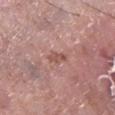Notes:
- biopsy status: total-body-photography surveillance lesion; no biopsy
- automated lesion analysis: a lesion color around L≈52 a*≈23 b*≈25 in CIELAB, roughly 9 lightness units darker than nearby skin, and a lesion-to-skin contrast of about 6.5 (normalized; higher = more distinct); a border-irregularity index near 3.5/10, a within-lesion color-variation index near 0.5/10, and radial color variation of about 0; a classifier nevus-likeness of about 0/100 and lesion-presence confidence of about 100/100
- lighting: white-light
- site: the right lower leg
- imaging modality: total-body-photography crop, ~15 mm field of view
- subject: male, aged 68 to 72
- lesion size: about 2.5 mm